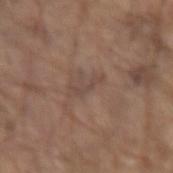The lesion was tiled from a total-body skin photograph and was not biopsied. Approximately 3.5 mm at its widest. The lesion is located on the arm. Cropped from a whole-body photographic skin survey; the tile spans about 15 mm. An algorithmic analysis of the crop reported an average lesion color of about L≈45 a*≈16 b*≈23 (CIELAB) and a lesion-to-skin contrast of about 5 (normalized; higher = more distinct). The software also gave an automated nevus-likeness rating near 0 out of 100 and lesion-presence confidence of about 95/100. A male patient, in their 80s.